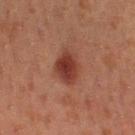Clinical impression:
No biopsy was performed on this lesion — it was imaged during a full skin examination and was not determined to be concerning.
Image and clinical context:
The lesion is located on the leg. The lesion's longest dimension is about 4 mm. The tile uses cross-polarized illumination. A region of skin cropped from a whole-body photographic capture, roughly 15 mm wide. A female patient, approximately 20 years of age.